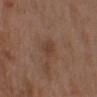biopsy status=catalogued during a skin exam; not biopsied
body site=the chest
automated lesion analysis=an automated nevus-likeness rating near 0 out of 100
diameter=≈2.5 mm
acquisition=~15 mm tile from a whole-body skin photo
patient=female, roughly 30 years of age